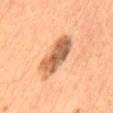Notes:
• notes: imaged on a skin check; not biopsied
• patient: male, in their mid-50s
• location: the abdomen
• size: ≈6 mm
• automated metrics: a footprint of about 14 mm² and an outline eccentricity of about 0.9 (0 = round, 1 = elongated); a lesion-detection confidence of about 100/100
• tile lighting: cross-polarized
• image: ~15 mm tile from a whole-body skin photo